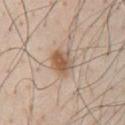<lesion>
  <biopsy_status>not biopsied; imaged during a skin examination</biopsy_status>
  <site>chest</site>
  <patient>
    <sex>male</sex>
    <age_approx>60</age_approx>
  </patient>
  <lighting>white-light</lighting>
  <automated_metrics>
    <area_mm2_approx>7.0</area_mm2_approx>
    <eccentricity>0.6</eccentricity>
    <shape_asymmetry>0.2</shape_asymmetry>
    <border_irregularity_0_10>2.0</border_irregularity_0_10>
    <color_variation_0_10>5.5</color_variation_0_10>
    <peripheral_color_asymmetry>1.5</peripheral_color_asymmetry>
    <nevus_likeness_0_100>95</nevus_likeness_0_100>
  </automated_metrics>
  <lesion_size>
    <long_diameter_mm_approx>3.5</long_diameter_mm_approx>
  </lesion_size>
  <image>
    <source>total-body photography crop</source>
    <field_of_view_mm>15</field_of_view_mm>
  </image>
</lesion>